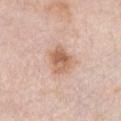Impression: The lesion was tiled from a total-body skin photograph and was not biopsied. Clinical summary: A female subject in their mid-60s. About 4 mm across. Captured under white-light illumination. Cropped from a whole-body photographic skin survey; the tile spans about 15 mm. Automated tile analysis of the lesion measured an area of roughly 8.5 mm², a shape eccentricity near 0.65, and a shape-asymmetry score of about 0.25 (0 = symmetric). The analysis additionally found a lesion–skin lightness drop of about 11 and a lesion-to-skin contrast of about 8 (normalized; higher = more distinct). The lesion is on the front of the torso.